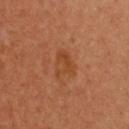This lesion was catalogued during total-body skin photography and was not selected for biopsy. A 15 mm close-up tile from a total-body photography series done for melanoma screening. This is a cross-polarized tile. The patient is a female about 50 years old.